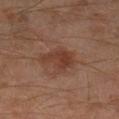Clinical impression: The lesion was photographed on a routine skin check and not biopsied; there is no pathology result. Image and clinical context: A male subject roughly 60 years of age. Longest diameter approximately 4 mm. A 15 mm close-up tile from a total-body photography series done for melanoma screening. On the left lower leg. Automated tile analysis of the lesion measured a lesion area of about 9.5 mm² and an eccentricity of roughly 0.55. And it measured a border-irregularity index near 4/10, a within-lesion color-variation index near 3/10, and peripheral color asymmetry of about 1. The software also gave a classifier nevus-likeness of about 20/100 and lesion-presence confidence of about 100/100. Imaged with cross-polarized lighting.